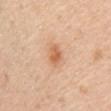biopsy status = imaged on a skin check; not biopsied | location = the chest | patient = female, in their mid-50s | image source = 15 mm crop, total-body photography | size = ≈2.5 mm | illumination = white-light.